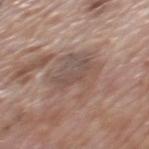This lesion was catalogued during total-body skin photography and was not selected for biopsy.
A 15 mm close-up extracted from a 3D total-body photography capture.
An algorithmic analysis of the crop reported an average lesion color of about L≈51 a*≈16 b*≈23 (CIELAB), about 8 CIELAB-L* units darker than the surrounding skin, and a lesion-to-skin contrast of about 6 (normalized; higher = more distinct).
The patient is a male aged around 70.
From the mid back.